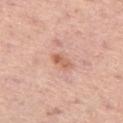Q: Was a biopsy performed?
A: catalogued during a skin exam; not biopsied
Q: Where on the body is the lesion?
A: the left thigh
Q: Patient demographics?
A: male, aged 73–77
Q: What is the imaging modality?
A: ~15 mm crop, total-body skin-cancer survey